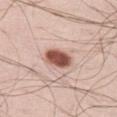Assessment: Imaged during a routine full-body skin examination; the lesion was not biopsied and no histopathology is available. Context: The lesion is on the leg. A 15 mm close-up extracted from a 3D total-body photography capture. An algorithmic analysis of the crop reported a lesion color around L≈54 a*≈23 b*≈26 in CIELAB and roughly 19 lightness units darker than nearby skin. The software also gave a border-irregularity index near 2/10, a color-variation rating of about 5/10, and a peripheral color-asymmetry measure near 2. A male patient aged 33 to 37. Measured at roughly 4 mm in maximum diameter.